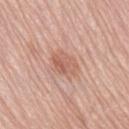biopsy status = total-body-photography surveillance lesion; no biopsy
patient = female, in their mid- to late 60s
acquisition = ~15 mm crop, total-body skin-cancer survey
body site = the lower back